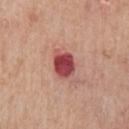Assessment: The lesion was tiled from a total-body skin photograph and was not biopsied. Background: From the chest. The patient is a male aged approximately 65. A 15 mm close-up tile from a total-body photography series done for melanoma screening. The recorded lesion diameter is about 4 mm. This is a white-light tile.